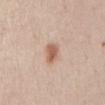This lesion was catalogued during total-body skin photography and was not selected for biopsy.
A female patient roughly 45 years of age.
On the abdomen.
Automated tile analysis of the lesion measured a lesion–skin lightness drop of about 12 and a normalized border contrast of about 8. The software also gave a border-irregularity rating of about 2.5/10, internal color variation of about 2.5 on a 0–10 scale, and a peripheral color-asymmetry measure near 0.5. The software also gave a classifier nevus-likeness of about 95/100 and a detector confidence of about 100 out of 100 that the crop contains a lesion.
The tile uses white-light illumination.
A close-up tile cropped from a whole-body skin photograph, about 15 mm across.